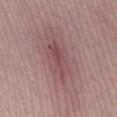The lesion was photographed on a routine skin check and not biopsied; there is no pathology result. The total-body-photography lesion software estimated an automated nevus-likeness rating near 0 out of 100. Measured at roughly 6 mm in maximum diameter. A 15 mm crop from a total-body photograph taken for skin-cancer surveillance. On the right lower leg. A female subject in their 60s. Captured under white-light illumination.